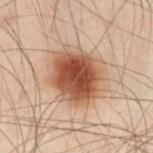follow-up = imaged on a skin check; not biopsied | lesion diameter = ≈6.5 mm | patient = male, roughly 50 years of age | lighting = cross-polarized | anatomic site = the left leg | image = 15 mm crop, total-body photography.